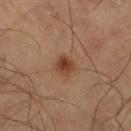Q: Was this lesion biopsied?
A: no biopsy performed (imaged during a skin exam)
Q: What kind of image is this?
A: 15 mm crop, total-body photography
Q: Where on the body is the lesion?
A: the left thigh
Q: What are the patient's age and sex?
A: male, in their mid-60s